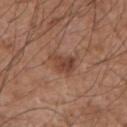Notes:
• patient: male, aged 58–62
• image-analysis metrics: a lesion color around L≈43 a*≈22 b*≈28 in CIELAB, about 10 CIELAB-L* units darker than the surrounding skin, and a normalized lesion–skin contrast near 7.5
• lighting: white-light
• location: the left upper arm
• image source: ~15 mm tile from a whole-body skin photo